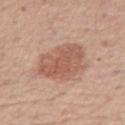The lesion was tiled from a total-body skin photograph and was not biopsied.